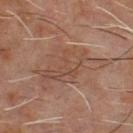<lesion>
  <biopsy_status>not biopsied; imaged during a skin examination</biopsy_status>
  <site>chest</site>
  <lighting>cross-polarized</lighting>
  <patient>
    <sex>male</sex>
    <age_approx>60</age_approx>
  </patient>
  <image>
    <source>total-body photography crop</source>
    <field_of_view_mm>15</field_of_view_mm>
  </image>
</lesion>